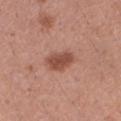workup — no biopsy performed (imaged during a skin exam)
image-analysis metrics — a lesion area of about 6.5 mm², a shape eccentricity near 0.8, and a symmetry-axis asymmetry near 0.2; about 12 CIELAB-L* units darker than the surrounding skin and a normalized lesion–skin contrast near 8.5; a border-irregularity index near 2/10, internal color variation of about 2.5 on a 0–10 scale, and a peripheral color-asymmetry measure near 1
lighting — white-light
subject — female, in their mid- to late 30s
image — ~15 mm tile from a whole-body skin photo
lesion diameter — ≈3.5 mm
body site — the right forearm